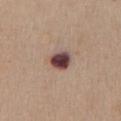workup = catalogued during a skin exam; not biopsied | body site = the chest | lighting = white-light | imaging modality = 15 mm crop, total-body photography | diameter = ≈2.5 mm | patient = male, aged 58 to 62.